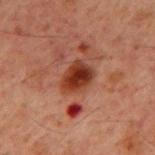Notes:
• follow-up — imaged on a skin check; not biopsied
• subject — male, aged 58 to 62
• lesion diameter — ~4 mm (longest diameter)
• image — 15 mm crop, total-body photography
• location — the mid back
• illumination — cross-polarized illumination